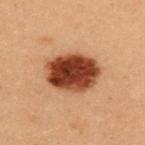On the upper back. A lesion tile, about 15 mm wide, cut from a 3D total-body photograph. The subject is a female aged 28–32.